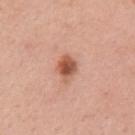No biopsy was performed on this lesion — it was imaged during a full skin examination and was not determined to be concerning. A roughly 15 mm field-of-view crop from a total-body skin photograph. The patient is a female in their mid-30s. Approximately 3 mm at its widest. The tile uses white-light illumination. From the left upper arm.